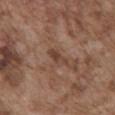Notes:
* follow-up: imaged on a skin check; not biopsied
* imaging modality: ~15 mm crop, total-body skin-cancer survey
* site: the chest
* patient: male, aged 73–77